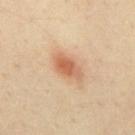| feature | finding |
|---|---|
| biopsy status | total-body-photography surveillance lesion; no biopsy |
| anatomic site | the mid back |
| lesion size | ~4 mm (longest diameter) |
| patient | male, aged approximately 50 |
| TBP lesion metrics | a footprint of about 7.5 mm² and a symmetry-axis asymmetry near 0.25; a mean CIELAB color near L≈62 a*≈22 b*≈35, roughly 11 lightness units darker than nearby skin, and a normalized lesion–skin contrast near 7.5 |
| imaging modality | ~15 mm crop, total-body skin-cancer survey |